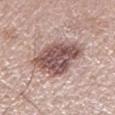Captured during whole-body skin photography for melanoma surveillance; the lesion was not biopsied. Approximately 7.5 mm at its widest. A lesion tile, about 15 mm wide, cut from a 3D total-body photograph. Captured under white-light illumination. The lesion is on the left lower leg. The patient is a male approximately 65 years of age.